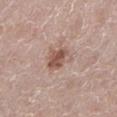Captured during whole-body skin photography for melanoma surveillance; the lesion was not biopsied. The lesion-visualizer software estimated a symmetry-axis asymmetry near 0.3. It also reported about 12 CIELAB-L* units darker than the surrounding skin. Cropped from a total-body skin-imaging series; the visible field is about 15 mm. A female patient about 50 years old. The tile uses white-light illumination. Located on the left lower leg. About 3.5 mm across.